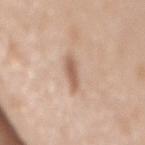biopsy status = imaged on a skin check; not biopsied | imaging modality = total-body-photography crop, ~15 mm field of view | tile lighting = white-light illumination | patient = female, in their 50s | size = ~3.5 mm (longest diameter) | location = the mid back | automated lesion analysis = a color-variation rating of about 1/10 and peripheral color asymmetry of about 0.